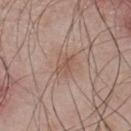Impression: The lesion was photographed on a routine skin check and not biopsied; there is no pathology result. Clinical summary: Located on the upper back. A male subject aged approximately 45. This image is a 15 mm lesion crop taken from a total-body photograph.